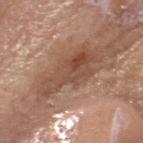image-analysis metrics=a footprint of about 16 mm², an eccentricity of roughly 0.95, and two-axis asymmetry of about 0.35; a lesion color around L≈48 a*≈20 b*≈29 in CIELAB and a normalized lesion–skin contrast near 8; peripheral color asymmetry of about 2 | image source=15 mm crop, total-body photography | patient=female, in their mid- to late 60s | body site=the head or neck | lesion diameter=~7.5 mm (longest diameter) | illumination=white-light.